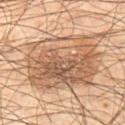| key | value |
|---|---|
| biopsy status | no biopsy performed (imaged during a skin exam) |
| patient | male, approximately 55 years of age |
| tile lighting | cross-polarized illumination |
| site | the left thigh |
| lesion size | about 9 mm |
| automated lesion analysis | a symmetry-axis asymmetry near 0.1; an average lesion color of about L≈54 a*≈18 b*≈31 (CIELAB), about 11 CIELAB-L* units darker than the surrounding skin, and a lesion-to-skin contrast of about 8 (normalized; higher = more distinct); border irregularity of about 2 on a 0–10 scale, a color-variation rating of about 8/10, and a peripheral color-asymmetry measure near 3; a detector confidence of about 100 out of 100 that the crop contains a lesion |
| image source | ~15 mm tile from a whole-body skin photo |